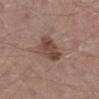This lesion was catalogued during total-body skin photography and was not selected for biopsy.